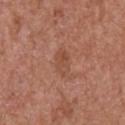No biopsy was performed on this lesion — it was imaged during a full skin examination and was not determined to be concerning. The lesion is on the right upper arm. The tile uses white-light illumination. About 3 mm across. Cropped from a whole-body photographic skin survey; the tile spans about 15 mm. A male subject, in their mid- to late 60s.